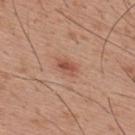Impression: Captured during whole-body skin photography for melanoma surveillance; the lesion was not biopsied. Clinical summary: Imaged with white-light lighting. Measured at roughly 2.5 mm in maximum diameter. An algorithmic analysis of the crop reported an eccentricity of roughly 0.75 and a shape-asymmetry score of about 0.15 (0 = symmetric). It also reported an average lesion color of about L≈53 a*≈24 b*≈30 (CIELAB), roughly 9 lightness units darker than nearby skin, and a lesion-to-skin contrast of about 6.5 (normalized; higher = more distinct). The software also gave border irregularity of about 1.5 on a 0–10 scale and a color-variation rating of about 3.5/10. A male patient, aged 28 to 32. From the back. A lesion tile, about 15 mm wide, cut from a 3D total-body photograph.